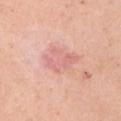No biopsy was performed on this lesion — it was imaged during a full skin examination and was not determined to be concerning. The lesion is located on the left upper arm. A female subject, about 65 years old. The lesion's longest dimension is about 4 mm. A 15 mm close-up extracted from a 3D total-body photography capture. The total-body-photography lesion software estimated a lesion-to-skin contrast of about 4.5 (normalized; higher = more distinct). The analysis additionally found a classifier nevus-likeness of about 0/100 and a detector confidence of about 100 out of 100 that the crop contains a lesion.